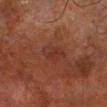follow-up = no biopsy performed (imaged during a skin exam)
subject = male, about 70 years old
site = the right lower leg
automated metrics = an area of roughly 5.5 mm², a shape eccentricity near 0.85, and a symmetry-axis asymmetry near 0.25; a color-variation rating of about 3/10 and peripheral color asymmetry of about 1; a nevus-likeness score of about 0/100 and a detector confidence of about 100 out of 100 that the crop contains a lesion
lighting = cross-polarized illumination
image source = total-body-photography crop, ~15 mm field of view
size = ≈3.5 mm